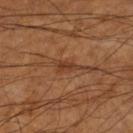<record>
  <biopsy_status>not biopsied; imaged during a skin examination</biopsy_status>
  <site>left lower leg</site>
  <patient>
    <sex>male</sex>
    <age_approx>60</age_approx>
  </patient>
  <lighting>cross-polarized</lighting>
  <image>
    <source>total-body photography crop</source>
    <field_of_view_mm>15</field_of_view_mm>
  </image>
</record>